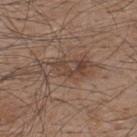Impression: Recorded during total-body skin imaging; not selected for excision or biopsy. Image and clinical context: A lesion tile, about 15 mm wide, cut from a 3D total-body photograph. Located on the upper back. The patient is a male approximately 45 years of age. Automated tile analysis of the lesion measured a footprint of about 11 mm² and a shape eccentricity near 0.9. It also reported a lesion–skin lightness drop of about 8 and a normalized border contrast of about 6.5. The software also gave an automated nevus-likeness rating near 5 out of 100 and a lesion-detection confidence of about 95/100.